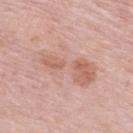No biopsy was performed on this lesion — it was imaged during a full skin examination and was not determined to be concerning.
Approximately 8 mm at its widest.
Located on the upper back.
A roughly 15 mm field-of-view crop from a total-body skin photograph.
A female subject, approximately 65 years of age.
Imaged with white-light lighting.